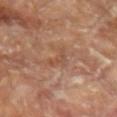Recorded during total-body skin imaging; not selected for excision or biopsy.
A roughly 15 mm field-of-view crop from a total-body skin photograph.
A male subject aged approximately 65.
On the left forearm.
Captured under cross-polarized illumination.